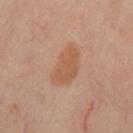On the right thigh.
An algorithmic analysis of the crop reported a lesion area of about 12 mm², an outline eccentricity of about 0.85 (0 = round, 1 = elongated), and two-axis asymmetry of about 0.25. And it measured a border-irregularity rating of about 2.5/10, internal color variation of about 2 on a 0–10 scale, and peripheral color asymmetry of about 0.5.
The patient is a female in their 50s.
Imaged with cross-polarized lighting.
A lesion tile, about 15 mm wide, cut from a 3D total-body photograph.